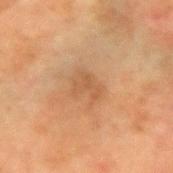Part of a total-body skin-imaging series; this lesion was reviewed on a skin check and was not flagged for biopsy.
Located on the arm.
Longest diameter approximately 3 mm.
A female patient approximately 55 years of age.
This image is a 15 mm lesion crop taken from a total-body photograph.
Captured under cross-polarized illumination.